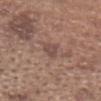biopsy_status: not biopsied; imaged during a skin examination
lesion_size:
  long_diameter_mm_approx: 2.5
lighting: white-light
site: head or neck
automated_metrics:
  border_irregularity_0_10: 3.5
  peripheral_color_asymmetry: 0.5
patient:
  sex: male
  age_approx: 65
image:
  source: total-body photography crop
  field_of_view_mm: 15